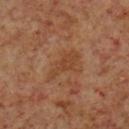Notes:
- follow-up: no biopsy performed (imaged during a skin exam)
- lesion size: ≈4.5 mm
- anatomic site: the left lower leg
- image source: 15 mm crop, total-body photography
- subject: male, approximately 60 years of age